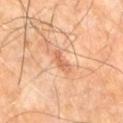Clinical impression:
No biopsy was performed on this lesion — it was imaged during a full skin examination and was not determined to be concerning.
Context:
A 15 mm crop from a total-body photograph taken for skin-cancer surveillance. A male patient aged approximately 60. From the right leg. This is a cross-polarized tile. About 2.5 mm across.